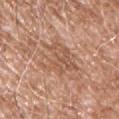Impression:
The lesion was tiled from a total-body skin photograph and was not biopsied.
Image and clinical context:
Automated image analysis of the tile measured an outline eccentricity of about 0.75 (0 = round, 1 = elongated) and a shape-asymmetry score of about 0.4 (0 = symmetric). The software also gave a lesion color around L≈56 a*≈21 b*≈31 in CIELAB and a lesion-to-skin contrast of about 6 (normalized; higher = more distinct). The analysis additionally found a nevus-likeness score of about 0/100. The lesion is on the chest. The subject is a male in their mid- to late 50s. A 15 mm close-up extracted from a 3D total-body photography capture.